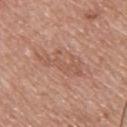Background: From the upper back. The subject is a male approximately 60 years of age. A 15 mm crop from a total-body photograph taken for skin-cancer surveillance.